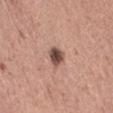follow-up: total-body-photography surveillance lesion; no biopsy | subject: female, aged 48 to 52 | site: the right thigh | imaging modality: ~15 mm tile from a whole-body skin photo | illumination: white-light | lesion diameter: about 2.5 mm.